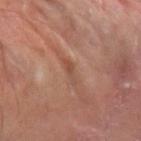Recorded during total-body skin imaging; not selected for excision or biopsy. A male subject, aged 68–72. Located on the left forearm. Automated tile analysis of the lesion measured an average lesion color of about L≈48 a*≈21 b*≈28 (CIELAB), roughly 7 lightness units darker than nearby skin, and a normalized border contrast of about 5.5. The software also gave border irregularity of about 4.5 on a 0–10 scale, a within-lesion color-variation index near 0.5/10, and radial color variation of about 0. Approximately 3 mm at its widest. This image is a 15 mm lesion crop taken from a total-body photograph. The tile uses cross-polarized illumination.